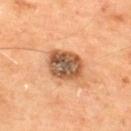workup: total-body-photography surveillance lesion; no biopsy | imaging modality: ~15 mm tile from a whole-body skin photo | lighting: cross-polarized | TBP lesion metrics: a footprint of about 12 mm², a shape eccentricity near 0.55, and a symmetry-axis asymmetry near 0.1; a classifier nevus-likeness of about 10/100 and a detector confidence of about 100 out of 100 that the crop contains a lesion | body site: the upper back | patient: male, in their mid-60s.